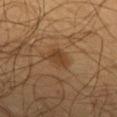Captured during whole-body skin photography for melanoma surveillance; the lesion was not biopsied. A roughly 15 mm field-of-view crop from a total-body skin photograph. Measured at roughly 3 mm in maximum diameter. The lesion is on the back. An algorithmic analysis of the crop reported a lesion area of about 4.5 mm², an outline eccentricity of about 0.75 (0 = round, 1 = elongated), and a symmetry-axis asymmetry near 0.3. The software also gave a lesion color around L≈36 a*≈18 b*≈31 in CIELAB, a lesion–skin lightness drop of about 7, and a normalized border contrast of about 7. Imaged with cross-polarized lighting. A male subject, approximately 55 years of age.